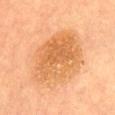Imaged during a routine full-body skin examination; the lesion was not biopsied and no histopathology is available. The recorded lesion diameter is about 7 mm. On the abdomen. A region of skin cropped from a whole-body photographic capture, roughly 15 mm wide. The patient is a female aged around 60. Imaged with cross-polarized lighting. Automated image analysis of the tile measured a classifier nevus-likeness of about 30/100 and lesion-presence confidence of about 100/100.